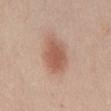Captured during whole-body skin photography for melanoma surveillance; the lesion was not biopsied.